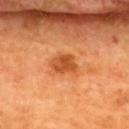Impression:
No biopsy was performed on this lesion — it was imaged during a full skin examination and was not determined to be concerning.
Background:
A 15 mm close-up tile from a total-body photography series done for melanoma screening. The lesion's longest dimension is about 3 mm. On the back. A female subject, aged approximately 45. This is a cross-polarized tile.